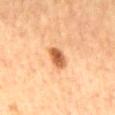Case summary:
* biopsy status — imaged on a skin check; not biopsied
* location — the mid back
* lesion diameter — ≈3 mm
* image-analysis metrics — a footprint of about 5 mm², an eccentricity of roughly 0.65, and two-axis asymmetry of about 0.15; a lesion color around L≈60 a*≈26 b*≈41 in CIELAB and a normalized lesion–skin contrast near 9.5; a classifier nevus-likeness of about 100/100 and a lesion-detection confidence of about 100/100
* patient — male, approximately 60 years of age
* image — ~15 mm crop, total-body skin-cancer survey
* lighting — cross-polarized illumination